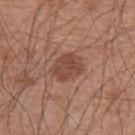Clinical impression:
This lesion was catalogued during total-body skin photography and was not selected for biopsy.
Image and clinical context:
Measured at roughly 4 mm in maximum diameter. The lesion is on the left upper arm. The total-body-photography lesion software estimated a footprint of about 9 mm², a shape eccentricity near 0.6, and two-axis asymmetry of about 0.2. And it measured a border-irregularity rating of about 2/10, internal color variation of about 2.5 on a 0–10 scale, and peripheral color asymmetry of about 1. A 15 mm crop from a total-body photograph taken for skin-cancer surveillance. The patient is a male aged around 55.